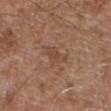follow-up: imaged on a skin check; not biopsied
illumination: white-light
anatomic site: the abdomen
lesion size: ~3.5 mm (longest diameter)
acquisition: ~15 mm tile from a whole-body skin photo
patient: male, aged 73 to 77